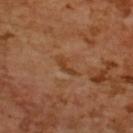Imaged during a routine full-body skin examination; the lesion was not biopsied and no histopathology is available. The lesion is located on the upper back. Automated image analysis of the tile measured a footprint of about 3 mm², an eccentricity of roughly 0.9, and a symmetry-axis asymmetry near 0.4. And it measured border irregularity of about 5 on a 0–10 scale. The patient is a female in their mid-50s. The recorded lesion diameter is about 3 mm. A 15 mm crop from a total-body photograph taken for skin-cancer surveillance.